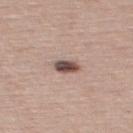{
  "biopsy_status": "not biopsied; imaged during a skin examination",
  "site": "upper back",
  "image": {
    "source": "total-body photography crop",
    "field_of_view_mm": 15
  },
  "automated_metrics": {
    "cielab_L": 48,
    "cielab_a": 17,
    "cielab_b": 21,
    "vs_skin_darker_L": 18.0,
    "vs_skin_contrast_norm": 12.5,
    "nevus_likeness_0_100": 90
  },
  "patient": {
    "sex": "female",
    "age_approx": 55
  },
  "lighting": "white-light"
}